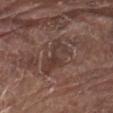Automated tile analysis of the lesion measured a lesion area of about 13 mm². The software also gave roughly 7 lightness units darker than nearby skin and a normalized border contrast of about 6. And it measured a border-irregularity index near 3.5/10 and internal color variation of about 5.5 on a 0–10 scale. Located on the chest. Captured under white-light illumination. Cropped from a whole-body photographic skin survey; the tile spans about 15 mm. The lesion's longest dimension is about 4.5 mm. A male patient, aged 78–82.